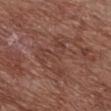Clinical impression: Captured during whole-body skin photography for melanoma surveillance; the lesion was not biopsied. Background: The total-body-photography lesion software estimated a lesion-to-skin contrast of about 4.5 (normalized; higher = more distinct). The software also gave a classifier nevus-likeness of about 0/100. On the chest. A female patient, aged approximately 80. A roughly 15 mm field-of-view crop from a total-body skin photograph.